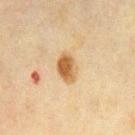Clinical impression: This lesion was catalogued during total-body skin photography and was not selected for biopsy. Acquisition and patient details: Automated tile analysis of the lesion measured an area of roughly 6.5 mm², an outline eccentricity of about 0.75 (0 = round, 1 = elongated), and two-axis asymmetry of about 0.2. The software also gave an average lesion color of about L≈50 a*≈16 b*≈35 (CIELAB). And it measured border irregularity of about 2 on a 0–10 scale, a color-variation rating of about 4.5/10, and peripheral color asymmetry of about 1.5. It also reported a classifier nevus-likeness of about 100/100. The lesion is located on the chest. This is a cross-polarized tile. A male patient, approximately 70 years of age. A roughly 15 mm field-of-view crop from a total-body skin photograph.